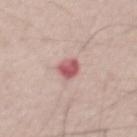Q: Was this lesion biopsied?
A: no biopsy performed (imaged during a skin exam)
Q: How was the tile lit?
A: white-light
Q: What are the patient's age and sex?
A: male, aged 53 to 57
Q: What kind of image is this?
A: 15 mm crop, total-body photography
Q: Lesion size?
A: ≈3 mm
Q: What did automated image analysis measure?
A: a lesion color around L≈57 a*≈28 b*≈21 in CIELAB, roughly 14 lightness units darker than nearby skin, and a normalized lesion–skin contrast near 9; a classifier nevus-likeness of about 0/100 and lesion-presence confidence of about 100/100